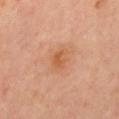Findings:
- workup · no biopsy performed (imaged during a skin exam)
- imaging modality · ~15 mm tile from a whole-body skin photo
- illumination · cross-polarized
- body site · the mid back
- patient · male, aged 48–52
- lesion diameter · ≈3 mm
- automated metrics · a mean CIELAB color near L≈57 a*≈24 b*≈37, roughly 7 lightness units darker than nearby skin, and a normalized lesion–skin contrast near 6; radial color variation of about 1; a nevus-likeness score of about 5/100 and a lesion-detection confidence of about 100/100